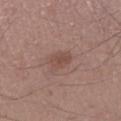notes: catalogued during a skin exam; not biopsied
acquisition: ~15 mm crop, total-body skin-cancer survey
anatomic site: the right lower leg
subject: male, about 55 years old